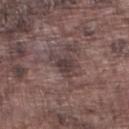Case summary:
- biopsy status · total-body-photography surveillance lesion; no biopsy
- image source · total-body-photography crop, ~15 mm field of view
- lighting · white-light illumination
- patient · male, in their mid-70s
- size · ≈3.5 mm
- image-analysis metrics · a mean CIELAB color near L≈38 a*≈15 b*≈15 and a lesion-to-skin contrast of about 7.5 (normalized; higher = more distinct)
- location · the left lower leg